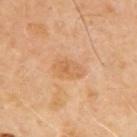Recorded during total-body skin imaging; not selected for excision or biopsy.
The lesion is located on the back.
A male subject, aged 68 to 72.
A roughly 15 mm field-of-view crop from a total-body skin photograph.